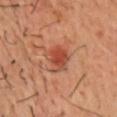Clinical impression: Recorded during total-body skin imaging; not selected for excision or biopsy. Image and clinical context: From the front of the torso. Measured at roughly 3.5 mm in maximum diameter. A 15 mm crop from a total-body photograph taken for skin-cancer surveillance. This is a cross-polarized tile. The total-body-photography lesion software estimated a footprint of about 8 mm², an eccentricity of roughly 0.5, and two-axis asymmetry of about 0.3. And it measured a lesion color around L≈49 a*≈30 b*≈34 in CIELAB and roughly 10 lightness units darker than nearby skin. A male patient aged 38 to 42.